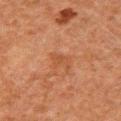Located on the arm. The total-body-photography lesion software estimated a lesion area of about 3.5 mm² and an outline eccentricity of about 0.85 (0 = round, 1 = elongated). The analysis additionally found a lesion color around L≈39 a*≈22 b*≈31 in CIELAB, a lesion–skin lightness drop of about 5, and a normalized border contrast of about 5. And it measured a within-lesion color-variation index near 0.5/10 and a peripheral color-asymmetry measure near 0. And it measured a classifier nevus-likeness of about 0/100 and a lesion-detection confidence of about 100/100. A lesion tile, about 15 mm wide, cut from a 3D total-body photograph. The tile uses cross-polarized illumination. About 3 mm across. A male patient, in their 60s.